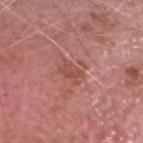This lesion was catalogued during total-body skin photography and was not selected for biopsy.
A male subject, approximately 75 years of age.
The lesion's longest dimension is about 3 mm.
The tile uses white-light illumination.
A 15 mm crop from a total-body photograph taken for skin-cancer surveillance.
The lesion-visualizer software estimated a footprint of about 4 mm² and an outline eccentricity of about 0.75 (0 = round, 1 = elongated). The software also gave a classifier nevus-likeness of about 0/100.
The lesion is on the head or neck.